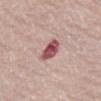notes = no biopsy performed (imaged during a skin exam) | tile lighting = white-light illumination | lesion diameter = about 3 mm | TBP lesion metrics = an average lesion color of about L≈52 a*≈28 b*≈19 (CIELAB), roughly 16 lightness units darker than nearby skin, and a normalized border contrast of about 11; a border-irregularity index near 2.5/10, a within-lesion color-variation index near 6/10, and radial color variation of about 2; a nevus-likeness score of about 0/100 and lesion-presence confidence of about 100/100 | subject = female, aged 63 to 67 | acquisition = total-body-photography crop, ~15 mm field of view | location = the abdomen.